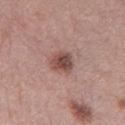biopsy_status: not biopsied; imaged during a skin examination
automated_metrics:
  area_mm2_approx: 5.5
  eccentricity: 0.35
  shape_asymmetry: 0.2
  vs_skin_darker_L: 13.0
  border_irregularity_0_10: 2.0
  color_variation_0_10: 5.0
  peripheral_color_asymmetry: 1.5
  nevus_likeness_0_100: 65
  lesion_detection_confidence_0_100: 100
image:
  source: total-body photography crop
  field_of_view_mm: 15
site: leg
lesion_size:
  long_diameter_mm_approx: 3.0
lighting: white-light
patient:
  sex: female
  age_approx: 40